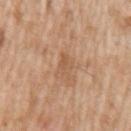follow-up — total-body-photography surveillance lesion; no biopsy
patient — male, in their mid- to late 60s
illumination — white-light illumination
imaging modality — ~15 mm crop, total-body skin-cancer survey
diameter — about 2.5 mm
image-analysis metrics — a lesion color around L≈56 a*≈20 b*≈34 in CIELAB, roughly 6 lightness units darker than nearby skin, and a normalized lesion–skin contrast near 4.5; an automated nevus-likeness rating near 0 out of 100
body site — the left upper arm